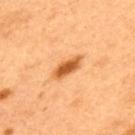* follow-up — imaged on a skin check; not biopsied
* image-analysis metrics — a border-irregularity index near 3.5/10 and radial color variation of about 0.5
* subject — male, approximately 50 years of age
* anatomic site — the upper back
* acquisition — total-body-photography crop, ~15 mm field of view